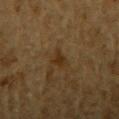{
  "biopsy_status": "not biopsied; imaged during a skin examination",
  "site": "right upper arm",
  "lighting": "cross-polarized",
  "lesion_size": {
    "long_diameter_mm_approx": 3.0
  },
  "image": {
    "source": "total-body photography crop",
    "field_of_view_mm": 15
  },
  "patient": {
    "sex": "male",
    "age_approx": "85+"
  }
}